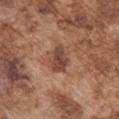Q: Was a biopsy performed?
A: imaged on a skin check; not biopsied
Q: What is the imaging modality?
A: ~15 mm crop, total-body skin-cancer survey
Q: What are the patient's age and sex?
A: male, aged 73–77
Q: Where on the body is the lesion?
A: the left upper arm